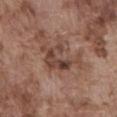Imaged during a routine full-body skin examination; the lesion was not biopsied and no histopathology is available.